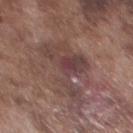The lesion was tiled from a total-body skin photograph and was not biopsied. Cropped from a total-body skin-imaging series; the visible field is about 15 mm. About 7.5 mm across. Located on the chest. The patient is a male in their mid-70s.